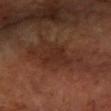workup: imaged on a skin check; not biopsied | image: ~15 mm crop, total-body skin-cancer survey | illumination: cross-polarized illumination | anatomic site: the left forearm | lesion size: about 3.5 mm | patient: female, about 70 years old | image-analysis metrics: a normalized border contrast of about 6; a border-irregularity index near 5.5/10, a color-variation rating of about 1.5/10, and a peripheral color-asymmetry measure near 0.5.